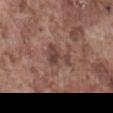notes = total-body-photography surveillance lesion; no biopsy
patient = male, aged 73–77
TBP lesion metrics = a lesion area of about 6 mm², an eccentricity of roughly 0.8, and a symmetry-axis asymmetry near 0.65; a mean CIELAB color near L≈43 a*≈18 b*≈22, about 8 CIELAB-L* units darker than the surrounding skin, and a normalized border contrast of about 7; a classifier nevus-likeness of about 0/100 and a lesion-detection confidence of about 100/100
anatomic site = the abdomen
illumination = white-light
image = total-body-photography crop, ~15 mm field of view
diameter = ≈4 mm